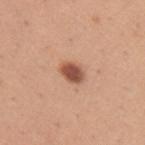Findings:
• notes: imaged on a skin check; not biopsied
• body site: the right upper arm
• image source: 15 mm crop, total-body photography
• automated metrics: a lesion color around L≈53 a*≈24 b*≈31 in CIELAB and roughly 15 lightness units darker than nearby skin; a border-irregularity index near 2/10, a color-variation rating of about 3.5/10, and radial color variation of about 1; an automated nevus-likeness rating near 100 out of 100 and lesion-presence confidence of about 100/100
• patient: female, aged around 30